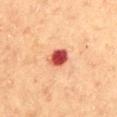Imaged during a routine full-body skin examination; the lesion was not biopsied and no histopathology is available. An algorithmic analysis of the crop reported an area of roughly 5 mm² and a shape eccentricity near 0.6. The patient is a male roughly 60 years of age. A 15 mm crop from a total-body photograph taken for skin-cancer surveillance. Approximately 3 mm at its widest. The lesion is on the abdomen.